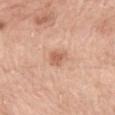Impression: Part of a total-body skin-imaging series; this lesion was reviewed on a skin check and was not flagged for biopsy. Clinical summary: The tile uses white-light illumination. This image is a 15 mm lesion crop taken from a total-body photograph. The recorded lesion diameter is about 2.5 mm. The lesion is located on the mid back. A female patient, in their 70s.